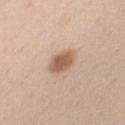biopsy_status: not biopsied; imaged during a skin examination
lesion_size:
  long_diameter_mm_approx: 3.5
image:
  source: total-body photography crop
  field_of_view_mm: 15
site: right upper arm
patient:
  sex: male
  age_approx: 30
lighting: white-light